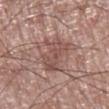The tile uses white-light illumination. From the right lower leg. A male patient, aged 68–72. A region of skin cropped from a whole-body photographic capture, roughly 15 mm wide. Automated image analysis of the tile measured a border-irregularity index near 6/10, internal color variation of about 3 on a 0–10 scale, and radial color variation of about 1. It also reported a lesion-detection confidence of about 100/100. Longest diameter approximately 5 mm.